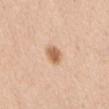Imaged during a routine full-body skin examination; the lesion was not biopsied and no histopathology is available.
Cropped from a total-body skin-imaging series; the visible field is about 15 mm.
A female subject, about 50 years old.
On the front of the torso.